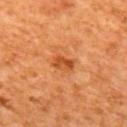notes = total-body-photography surveillance lesion; no biopsy
anatomic site = the mid back
automated metrics = roughly 10 lightness units darker than nearby skin and a normalized lesion–skin contrast near 7.5
patient = male, aged approximately 65
image = total-body-photography crop, ~15 mm field of view
diameter = ≈2.5 mm